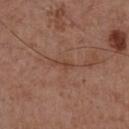{"biopsy_status": "not biopsied; imaged during a skin examination"}